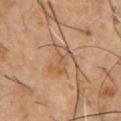{
  "biopsy_status": "not biopsied; imaged during a skin examination",
  "lighting": "cross-polarized",
  "image": {
    "source": "total-body photography crop",
    "field_of_view_mm": 15
  },
  "lesion_size": {
    "long_diameter_mm_approx": 3.5
  },
  "patient": {
    "sex": "male",
    "age_approx": 55
  },
  "site": "chest"
}